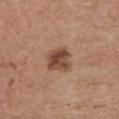| field | value |
|---|---|
| biopsy status | catalogued during a skin exam; not biopsied |
| subject | female, in their mid- to late 60s |
| site | the chest |
| lighting | white-light |
| acquisition | 15 mm crop, total-body photography |